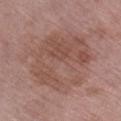{"biopsy_status": "not biopsied; imaged during a skin examination", "image": {"source": "total-body photography crop", "field_of_view_mm": 15}, "patient": {"sex": "female", "age_approx": 70}, "lighting": "white-light", "automated_metrics": {"cielab_L": 51, "cielab_a": 20, "cielab_b": 24, "vs_skin_darker_L": 7.0, "border_irregularity_0_10": 3.5, "peripheral_color_asymmetry": 2.0}, "lesion_size": {"long_diameter_mm_approx": 8.5}, "site": "right lower leg"}